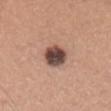body site = the lower back
subject = male, aged around 30
illumination = white-light illumination
size = about 3.5 mm
image source = 15 mm crop, total-body photography